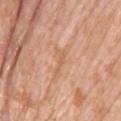notes: no biopsy performed (imaged during a skin exam) | lesion diameter: ≈3.5 mm | imaging modality: ~15 mm crop, total-body skin-cancer survey | lighting: white-light | patient: male, aged approximately 75 | site: the upper back.